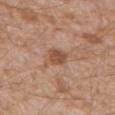Impression:
This lesion was catalogued during total-body skin photography and was not selected for biopsy.
Background:
A roughly 15 mm field-of-view crop from a total-body skin photograph. From the back. The subject is a male aged 58 to 62.